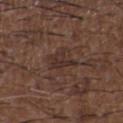{"biopsy_status": "not biopsied; imaged during a skin examination", "image": {"source": "total-body photography crop", "field_of_view_mm": 15}, "patient": {"sex": "male", "age_approx": 50}, "lighting": "white-light", "site": "upper back", "lesion_size": {"long_diameter_mm_approx": 4.0}}